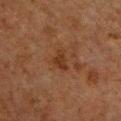Impression:
Recorded during total-body skin imaging; not selected for excision or biopsy.
Background:
From the chest. A close-up tile cropped from a whole-body skin photograph, about 15 mm across. The subject is a male about 60 years old.